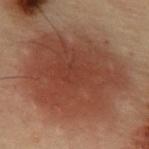Impression:
Imaged during a routine full-body skin examination; the lesion was not biopsied and no histopathology is available.
Image and clinical context:
An algorithmic analysis of the crop reported an area of roughly 75 mm², an eccentricity of roughly 0.55, and a symmetry-axis asymmetry near 0.15. The analysis additionally found a detector confidence of about 100 out of 100 that the crop contains a lesion. A male patient, approximately 55 years of age. About 11.5 mm across. A lesion tile, about 15 mm wide, cut from a 3D total-body photograph. This is a cross-polarized tile. From the abdomen.